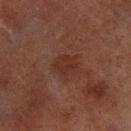This lesion was catalogued during total-body skin photography and was not selected for biopsy.
The subject is a male aged approximately 70.
The total-body-photography lesion software estimated a footprint of about 5 mm², a shape eccentricity near 0.5, and a symmetry-axis asymmetry near 0.2. The analysis additionally found an average lesion color of about L≈24 a*≈19 b*≈21 (CIELAB) and roughly 5 lightness units darker than nearby skin.
Captured under cross-polarized illumination.
The lesion is located on the left lower leg.
A region of skin cropped from a whole-body photographic capture, roughly 15 mm wide.
Approximately 2.5 mm at its widest.